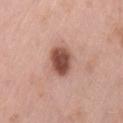The lesion is located on the lower back. Captured under white-light illumination. Automated tile analysis of the lesion measured a border-irregularity rating of about 1.5/10 and peripheral color asymmetry of about 1.5. And it measured a lesion-detection confidence of about 100/100. This image is a 15 mm lesion crop taken from a total-body photograph. Approximately 3.5 mm at its widest. The patient is a male in their mid-50s.